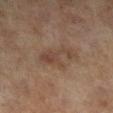Assessment:
Captured during whole-body skin photography for melanoma surveillance; the lesion was not biopsied.
Image and clinical context:
From the leg. A 15 mm close-up extracted from a 3D total-body photography capture. A female patient aged approximately 60. Longest diameter approximately 4.5 mm.